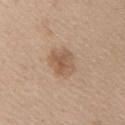Captured during whole-body skin photography for melanoma surveillance; the lesion was not biopsied. This image is a 15 mm lesion crop taken from a total-body photograph. The lesion is located on the arm. A female subject in their mid-20s.